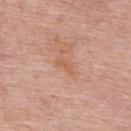<record>
<biopsy_status>not biopsied; imaged during a skin examination</biopsy_status>
<image>
  <source>total-body photography crop</source>
  <field_of_view_mm>15</field_of_view_mm>
</image>
<patient>
  <sex>male</sex>
  <age_approx>75</age_approx>
</patient>
<lighting>white-light</lighting>
<lesion_size>
  <long_diameter_mm_approx>2.5</long_diameter_mm_approx>
</lesion_size>
<site>upper back</site>
</record>